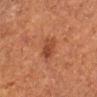A roughly 15 mm field-of-view crop from a total-body skin photograph.
A female subject in their 50s.
The recorded lesion diameter is about 3 mm.
The lesion is located on the left forearm.
The tile uses cross-polarized illumination.
The lesion-visualizer software estimated a nevus-likeness score of about 30/100 and lesion-presence confidence of about 100/100.